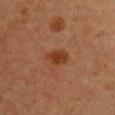Assessment: Recorded during total-body skin imaging; not selected for excision or biopsy. Acquisition and patient details: Captured under cross-polarized illumination. From the arm. Measured at roughly 3 mm in maximum diameter. A female subject aged around 55. A 15 mm close-up extracted from a 3D total-body photography capture.